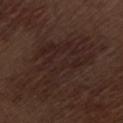Assessment: Part of a total-body skin-imaging series; this lesion was reviewed on a skin check and was not flagged for biopsy. Background: The lesion is located on the leg. A male subject roughly 70 years of age. The recorded lesion diameter is about 9 mm. An algorithmic analysis of the crop reported an average lesion color of about L≈22 a*≈15 b*≈18 (CIELAB) and a normalized border contrast of about 5. A lesion tile, about 15 mm wide, cut from a 3D total-body photograph. Imaged with white-light lighting.